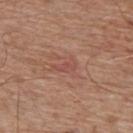Notes:
• biopsy status: no biopsy performed (imaged during a skin exam)
• image source: ~15 mm tile from a whole-body skin photo
• site: the back
• subject: male, in their mid-60s